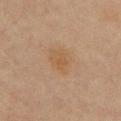notes = total-body-photography surveillance lesion; no biopsy
imaging modality = ~15 mm tile from a whole-body skin photo
subject = female, roughly 45 years of age
location = the chest